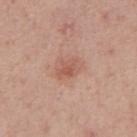Notes:
– biopsy status — catalogued during a skin exam; not biopsied
– TBP lesion metrics — an area of roughly 4.5 mm², a shape eccentricity near 0.8, and two-axis asymmetry of about 0.25; a lesion color around L≈56 a*≈23 b*≈28 in CIELAB, roughly 8 lightness units darker than nearby skin, and a normalized lesion–skin contrast near 6; internal color variation of about 3 on a 0–10 scale and peripheral color asymmetry of about 1; a nevus-likeness score of about 50/100 and a detector confidence of about 100 out of 100 that the crop contains a lesion
– image — total-body-photography crop, ~15 mm field of view
– anatomic site — the front of the torso
– tile lighting — white-light illumination
– subject — male, aged around 50